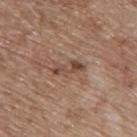image:
  source: total-body photography crop
  field_of_view_mm: 15
patient:
  sex: male
  age_approx: 70
lesion_size:
  long_diameter_mm_approx: 3.5
site: upper back